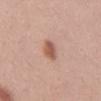The lesion was photographed on a routine skin check and not biopsied; there is no pathology result. A region of skin cropped from a whole-body photographic capture, roughly 15 mm wide. A female patient, roughly 35 years of age. About 4 mm across. Imaged with white-light lighting. Automated tile analysis of the lesion measured a lesion-detection confidence of about 100/100. Located on the mid back.